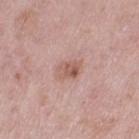Captured during whole-body skin photography for melanoma surveillance; the lesion was not biopsied.
Approximately 2.5 mm at its widest.
A female patient aged approximately 40.
Located on the left thigh.
Captured under white-light illumination.
A lesion tile, about 15 mm wide, cut from a 3D total-body photograph.